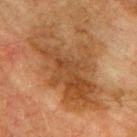follow-up: imaged on a skin check; not biopsied
image: ~15 mm crop, total-body skin-cancer survey
body site: the back
diameter: ≈11 mm
subject: male, aged 73–77